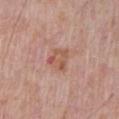Notes:
• site: the chest
• patient: male, approximately 70 years of age
• imaging modality: total-body-photography crop, ~15 mm field of view
• illumination: white-light illumination
• lesion diameter: about 3 mm